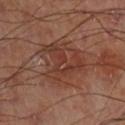Image and clinical context:
A 15 mm crop from a total-body photograph taken for skin-cancer surveillance. Approximately 6 mm at its widest. The total-body-photography lesion software estimated an average lesion color of about L≈39 a*≈23 b*≈27 (CIELAB), about 7 CIELAB-L* units darker than the surrounding skin, and a normalized border contrast of about 6. Captured under cross-polarized illumination. From the right thigh.